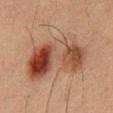biopsy_status: not biopsied; imaged during a skin examination
patient:
  sex: male
  age_approx: 50
image:
  source: total-body photography crop
  field_of_view_mm: 15
site: chest